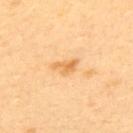follow-up: total-body-photography surveillance lesion; no biopsy
imaging modality: ~15 mm tile from a whole-body skin photo
body site: the upper back
patient: male, aged 38–42
tile lighting: cross-polarized illumination
size: about 3 mm